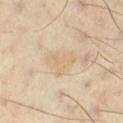| key | value |
|---|---|
| notes | no biopsy performed (imaged during a skin exam) |
| acquisition | ~15 mm crop, total-body skin-cancer survey |
| subject | male, aged around 55 |
| location | the left lower leg |
| lesion diameter | about 4 mm |